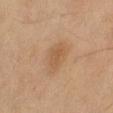Q: Is there a histopathology result?
A: catalogued during a skin exam; not biopsied
Q: What are the patient's age and sex?
A: female, approximately 60 years of age
Q: How was this image acquired?
A: ~15 mm crop, total-body skin-cancer survey
Q: What is the anatomic site?
A: the right thigh
Q: What did automated image analysis measure?
A: a mean CIELAB color near L≈49 a*≈18 b*≈32, roughly 7 lightness units darker than nearby skin, and a lesion-to-skin contrast of about 5.5 (normalized; higher = more distinct); a nevus-likeness score of about 35/100